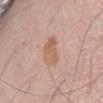Background:
The lesion is on the front of the torso. A region of skin cropped from a whole-body photographic capture, roughly 15 mm wide. The patient is a male aged around 60.